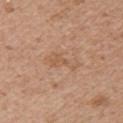size: about 4 mm
location: the arm
tile lighting: white-light
image-analysis metrics: a color-variation rating of about 1/10 and peripheral color asymmetry of about 0.5; an automated nevus-likeness rating near 0 out of 100 and a lesion-detection confidence of about 100/100
image source: ~15 mm crop, total-body skin-cancer survey
subject: female, aged around 60